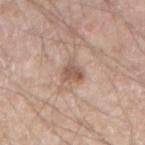Clinical impression: This lesion was catalogued during total-body skin photography and was not selected for biopsy. Background: The subject is a male aged 43 to 47. Cropped from a whole-body photographic skin survey; the tile spans about 15 mm. Longest diameter approximately 3 mm. The tile uses white-light illumination. The lesion is on the right forearm.